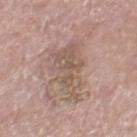No biopsy was performed on this lesion — it was imaged during a full skin examination and was not determined to be concerning.
The recorded lesion diameter is about 7 mm.
A male subject aged around 80.
A roughly 15 mm field-of-view crop from a total-body skin photograph.
From the right thigh.